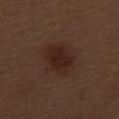biopsy_status: not biopsied; imaged during a skin examination
lesion_size:
  long_diameter_mm_approx: 4.5
site: left thigh
image:
  source: total-body photography crop
  field_of_view_mm: 15
lighting: white-light
patient:
  sex: male
  age_approx: 70
automated_metrics:
  border_irregularity_0_10: 2.0
  peripheral_color_asymmetry: 1.0
  nevus_likeness_0_100: 85
  lesion_detection_confidence_0_100: 100